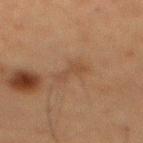Clinical summary: The lesion is located on the back. The recorded lesion diameter is about 3.5 mm. A roughly 15 mm field-of-view crop from a total-body skin photograph. Captured under cross-polarized illumination. A male patient in their 60s. The total-body-photography lesion software estimated a lesion area of about 4 mm², an outline eccentricity of about 0.9 (0 = round, 1 = elongated), and two-axis asymmetry of about 0.45. And it measured an average lesion color of about L≈36 a*≈15 b*≈26 (CIELAB) and a lesion–skin lightness drop of about 5. And it measured a nevus-likeness score of about 0/100.